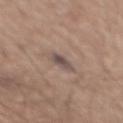The lesion was photographed on a routine skin check and not biopsied; there is no pathology result. A 15 mm crop from a total-body photograph taken for skin-cancer surveillance. Captured under white-light illumination. About 3 mm across. A male patient roughly 65 years of age. From the mid back.